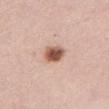| feature | finding |
|---|---|
| workup | total-body-photography surveillance lesion; no biopsy |
| body site | the chest |
| size | about 3 mm |
| automated metrics | an eccentricity of roughly 0.55 and a symmetry-axis asymmetry near 0.15; a lesion–skin lightness drop of about 17 and a normalized lesion–skin contrast near 11; a nevus-likeness score of about 100/100 and a lesion-detection confidence of about 100/100 |
| image | total-body-photography crop, ~15 mm field of view |
| illumination | white-light |
| subject | female, aged 48 to 52 |